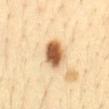biopsy status: imaged on a skin check; not biopsied | image: ~15 mm crop, total-body skin-cancer survey | site: the abdomen | patient: male, aged 33–37.